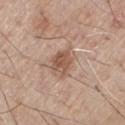Impression: No biopsy was performed on this lesion — it was imaged during a full skin examination and was not determined to be concerning. Clinical summary: A male patient aged around 80. An algorithmic analysis of the crop reported a lesion area of about 6 mm², an eccentricity of roughly 0.6, and two-axis asymmetry of about 0.2. And it measured an automated nevus-likeness rating near 45 out of 100 and a detector confidence of about 100 out of 100 that the crop contains a lesion. The recorded lesion diameter is about 3.5 mm. A 15 mm close-up extracted from a 3D total-body photography capture. This is a white-light tile. On the chest.